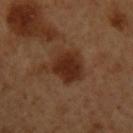Background:
The lesion's longest dimension is about 5.5 mm. Automated tile analysis of the lesion measured a lesion area of about 13 mm², an outline eccentricity of about 0.6 (0 = round, 1 = elongated), and a shape-asymmetry score of about 0.4 (0 = symmetric). It also reported a border-irregularity rating of about 4.5/10 and a within-lesion color-variation index near 2.5/10. The tile uses cross-polarized illumination. Located on the left upper arm. A 15 mm crop from a total-body photograph taken for skin-cancer surveillance. A male subject in their 50s.